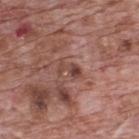subject: male, aged 68–72
site: the upper back
image: 15 mm crop, total-body photography